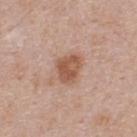<case>
<biopsy_status>not biopsied; imaged during a skin examination</biopsy_status>
<image>
  <source>total-body photography crop</source>
  <field_of_view_mm>15</field_of_view_mm>
</image>
<site>upper back</site>
<patient>
  <sex>male</sex>
  <age_approx>45</age_approx>
</patient>
</case>